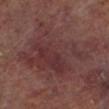Imaged during a routine full-body skin examination; the lesion was not biopsied and no histopathology is available. The tile uses cross-polarized illumination. On the left lower leg. A region of skin cropped from a whole-body photographic capture, roughly 15 mm wide. The lesion-visualizer software estimated a border-irregularity rating of about 6.5/10 and a color-variation rating of about 4.5/10. The lesion's longest dimension is about 9.5 mm. A male patient, aged 63 to 67.